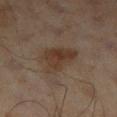Findings:
• workup: no biopsy performed (imaged during a skin exam)
• acquisition: ~15 mm tile from a whole-body skin photo
• lighting: cross-polarized illumination
• patient: male, about 65 years old
• site: the right lower leg